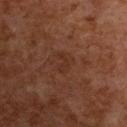follow-up: catalogued during a skin exam; not biopsied
image: ~15 mm tile from a whole-body skin photo
illumination: cross-polarized
subject: female, approximately 60 years of age
site: the upper back